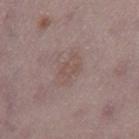Notes:
* follow-up: total-body-photography surveillance lesion; no biopsy
* image source: total-body-photography crop, ~15 mm field of view
* TBP lesion metrics: an area of roughly 5.5 mm²; a mean CIELAB color near L≈51 a*≈16 b*≈21, about 5 CIELAB-L* units darker than the surrounding skin, and a lesion-to-skin contrast of about 4.5 (normalized; higher = more distinct); border irregularity of about 3 on a 0–10 scale and radial color variation of about 0.5; a nevus-likeness score of about 0/100 and a lesion-detection confidence of about 100/100
* illumination: white-light illumination
* diameter: ≈3.5 mm
* patient: female, aged 48–52
* location: the right thigh